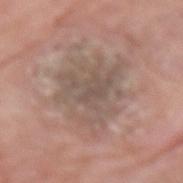biopsy status: total-body-photography surveillance lesion; no biopsy | body site: the left forearm | patient: male, aged 78 to 82 | acquisition: total-body-photography crop, ~15 mm field of view | lighting: white-light illumination.